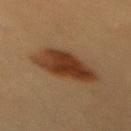notes: no biopsy performed (imaged during a skin exam) | site: the mid back | lesion size: ~6 mm (longest diameter) | imaging modality: 15 mm crop, total-body photography | tile lighting: cross-polarized illumination | patient: female, approximately 20 years of age.